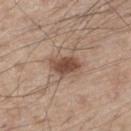biopsy status = imaged on a skin check; not biopsied
image = ~15 mm tile from a whole-body skin photo
illumination = white-light illumination
patient = male, in their 60s
site = the left thigh
size = ≈4 mm